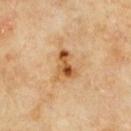biopsy status = catalogued during a skin exam; not biopsied
subject = male, aged 58 to 62
diameter = ≈4 mm
body site = the mid back
lighting = cross-polarized illumination
acquisition = ~15 mm tile from a whole-body skin photo